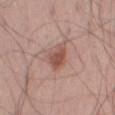– automated metrics — a border-irregularity rating of about 3/10, internal color variation of about 2.5 on a 0–10 scale, and a peripheral color-asymmetry measure near 1; a nevus-likeness score of about 75/100 and a detector confidence of about 100 out of 100 that the crop contains a lesion
– patient — male, aged 38–42
– site — the left thigh
– imaging modality — total-body-photography crop, ~15 mm field of view